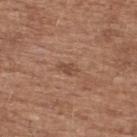The lesion was photographed on a routine skin check and not biopsied; there is no pathology result. On the upper back. An algorithmic analysis of the crop reported a border-irregularity index near 2.5/10, internal color variation of about 1 on a 0–10 scale, and a peripheral color-asymmetry measure near 0. And it measured a classifier nevus-likeness of about 5/100 and lesion-presence confidence of about 100/100. Cropped from a whole-body photographic skin survey; the tile spans about 15 mm. Measured at roughly 2.5 mm in maximum diameter. The subject is a male in their mid-70s. This is a white-light tile.